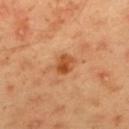Findings:
* imaging modality · ~15 mm tile from a whole-body skin photo
* body site · the mid back
* subject · male, aged around 45
* lighting · cross-polarized